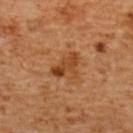biopsy status: catalogued during a skin exam; not biopsied | patient: female, aged 53 to 57 | lighting: cross-polarized | image source: ~15 mm tile from a whole-body skin photo | automated lesion analysis: a lesion–skin lightness drop of about 10 and a normalized border contrast of about 8; a color-variation rating of about 5.5/10 and a peripheral color-asymmetry measure near 1.5 | diameter: ≈3.5 mm | anatomic site: the upper back.